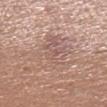biopsy status: imaged on a skin check; not biopsied
location: the left lower leg
subject: male, in their 40s
TBP lesion metrics: a lesion area of about 18 mm² and a shape eccentricity near 0.85; a mean CIELAB color near L≈58 a*≈18 b*≈24, roughly 6 lightness units darker than nearby skin, and a normalized border contrast of about 4.5; a peripheral color-asymmetry measure near 2; a nevus-likeness score of about 0/100 and lesion-presence confidence of about 80/100
imaging modality: ~15 mm crop, total-body skin-cancer survey
lighting: white-light
diameter: ≈7 mm